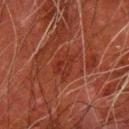Part of a total-body skin-imaging series; this lesion was reviewed on a skin check and was not flagged for biopsy.
About 4 mm across.
The patient is a male approximately 80 years of age.
From the left forearm.
Cropped from a total-body skin-imaging series; the visible field is about 15 mm.
Captured under cross-polarized illumination.